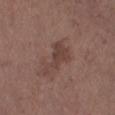This lesion was catalogued during total-body skin photography and was not selected for biopsy. Longest diameter approximately 5 mm. The lesion is on the left lower leg. Cropped from a whole-body photographic skin survey; the tile spans about 15 mm. The subject is a male aged approximately 75.